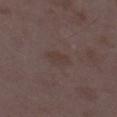Captured during whole-body skin photography for melanoma surveillance; the lesion was not biopsied.
The lesion's longest dimension is about 3.5 mm.
The lesion-visualizer software estimated an area of roughly 5.5 mm² and an eccentricity of roughly 0.85. It also reported a border-irregularity rating of about 2.5/10, a color-variation rating of about 2/10, and peripheral color asymmetry of about 0.5.
A female patient aged 33 to 37.
This image is a 15 mm lesion crop taken from a total-body photograph.
On the left thigh.